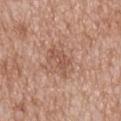notes: catalogued during a skin exam; not biopsied
patient: male, aged around 65
lighting: white-light illumination
location: the mid back
image source: 15 mm crop, total-body photography